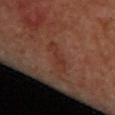Assessment: Part of a total-body skin-imaging series; this lesion was reviewed on a skin check and was not flagged for biopsy. Acquisition and patient details: A female subject, roughly 40 years of age. The lesion is on the upper back. Longest diameter approximately 4 mm. This image is a 15 mm lesion crop taken from a total-body photograph. The tile uses cross-polarized illumination.